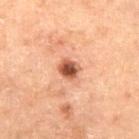Q: Was this lesion biopsied?
A: imaged on a skin check; not biopsied
Q: What kind of image is this?
A: ~15 mm tile from a whole-body skin photo
Q: Patient demographics?
A: male, aged around 70
Q: Illumination type?
A: cross-polarized
Q: What is the lesion's diameter?
A: ≈2.5 mm
Q: Where on the body is the lesion?
A: the abdomen
Q: What did automated image analysis measure?
A: a nevus-likeness score of about 95/100 and lesion-presence confidence of about 100/100